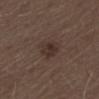{"patient": {"sex": "male", "age_approx": 70}, "image": {"source": "total-body photography crop", "field_of_view_mm": 15}, "site": "left thigh", "lighting": "white-light"}